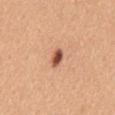workup — catalogued during a skin exam; not biopsied
subject — female, aged 43–47
tile lighting — white-light illumination
TBP lesion metrics — border irregularity of about 1.5 on a 0–10 scale, a color-variation rating of about 5/10, and peripheral color asymmetry of about 1.5
body site — the mid back
image — total-body-photography crop, ~15 mm field of view